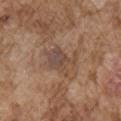{
  "biopsy_status": "not biopsied; imaged during a skin examination",
  "site": "right upper arm",
  "image": {
    "source": "total-body photography crop",
    "field_of_view_mm": 15
  },
  "patient": {
    "sex": "male",
    "age_approx": 75
  },
  "lighting": "white-light"
}